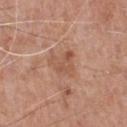Part of a total-body skin-imaging series; this lesion was reviewed on a skin check and was not flagged for biopsy. A male subject, roughly 70 years of age. Measured at roughly 4 mm in maximum diameter. Cropped from a whole-body photographic skin survey; the tile spans about 15 mm. This is a white-light tile. The lesion is on the front of the torso.